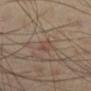The lesion was photographed on a routine skin check and not biopsied; there is no pathology result. The lesion is on the leg. Automated image analysis of the tile measured a border-irregularity index near 3.5/10, internal color variation of about 0 on a 0–10 scale, and a peripheral color-asymmetry measure near 0. The software also gave lesion-presence confidence of about 95/100. A 15 mm close-up extracted from a 3D total-body photography capture. The patient is a male aged 53–57. The recorded lesion diameter is about 2.5 mm.